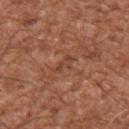Part of a total-body skin-imaging series; this lesion was reviewed on a skin check and was not flagged for biopsy.
On the chest.
A 15 mm close-up tile from a total-body photography series done for melanoma screening.
A male patient about 45 years old.
Automated image analysis of the tile measured a footprint of about 2.5 mm², an outline eccentricity of about 0.9 (0 = round, 1 = elongated), and a shape-asymmetry score of about 0.45 (0 = symmetric). The analysis additionally found border irregularity of about 6.5 on a 0–10 scale, internal color variation of about 0 on a 0–10 scale, and a peripheral color-asymmetry measure near 0. The analysis additionally found a lesion-detection confidence of about 95/100.